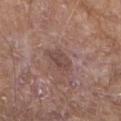{
  "biopsy_status": "not biopsied; imaged during a skin examination",
  "lighting": "white-light",
  "site": "right upper arm",
  "image": {
    "source": "total-body photography crop",
    "field_of_view_mm": 15
  },
  "patient": {
    "sex": "male",
    "age_approx": "85+"
  },
  "automated_metrics": {
    "border_irregularity_0_10": 2.5,
    "color_variation_0_10": 2.0,
    "peripheral_color_asymmetry": 1.0
  },
  "lesion_size": {
    "long_diameter_mm_approx": 3.0
  }
}